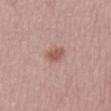workup: catalogued during a skin exam; not biopsied | anatomic site: the right lower leg | lesion diameter: ~2.5 mm (longest diameter) | tile lighting: white-light illumination | patient: female, in their 50s | imaging modality: ~15 mm tile from a whole-body skin photo.